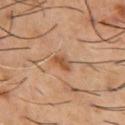workup: total-body-photography surveillance lesion; no biopsy | lesion diameter: ~2.5 mm (longest diameter) | patient: male, in their mid- to late 50s | illumination: cross-polarized | image source: total-body-photography crop, ~15 mm field of view | location: the front of the torso | image-analysis metrics: an area of roughly 4 mm², an outline eccentricity of about 0.75 (0 = round, 1 = elongated), and a symmetry-axis asymmetry near 0.25; an average lesion color of about L≈51 a*≈22 b*≈35 (CIELAB), a lesion–skin lightness drop of about 10, and a normalized lesion–skin contrast near 7.5.